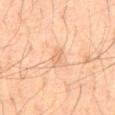Impression: The lesion was tiled from a total-body skin photograph and was not biopsied. Clinical summary: About 3 mm across. Cropped from a whole-body photographic skin survey; the tile spans about 15 mm. An algorithmic analysis of the crop reported an average lesion color of about L≈58 a*≈18 b*≈31 (CIELAB), about 7 CIELAB-L* units darker than the surrounding skin, and a normalized lesion–skin contrast near 4.5. It also reported a border-irregularity index near 3/10 and a within-lesion color-variation index near 1/10. A male subject, roughly 70 years of age. Captured under cross-polarized illumination. From the abdomen.